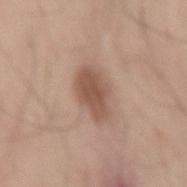notes: no biopsy performed (imaged during a skin exam)
image-analysis metrics: a color-variation rating of about 2/10 and a peripheral color-asymmetry measure near 0.5; lesion-presence confidence of about 100/100
site: the right thigh
subject: male, aged approximately 70
image source: 15 mm crop, total-body photography
size: ~4.5 mm (longest diameter)
tile lighting: white-light